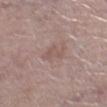- notes — imaged on a skin check; not biopsied
- tile lighting — white-light illumination
- lesion size — ~3 mm (longest diameter)
- subject — female, aged 63 to 67
- location — the leg
- image — ~15 mm crop, total-body skin-cancer survey